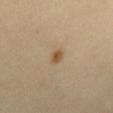Captured under cross-polarized illumination. Located on the back. The total-body-photography lesion software estimated a mean CIELAB color near L≈54 a*≈16 b*≈35, a lesion–skin lightness drop of about 10, and a lesion-to-skin contrast of about 8 (normalized; higher = more distinct). And it measured a nevus-likeness score of about 95/100 and lesion-presence confidence of about 100/100. The recorded lesion diameter is about 2 mm. A lesion tile, about 15 mm wide, cut from a 3D total-body photograph. A female patient approximately 60 years of age.